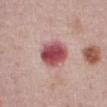biopsy_status: not biopsied; imaged during a skin examination
patient:
  sex: male
  age_approx: 75
image:
  source: total-body photography crop
  field_of_view_mm: 15
automated_metrics:
  cielab_L: 51
  cielab_a: 31
  cielab_b: 20
  vs_skin_darker_L: 17.0
site: abdomen